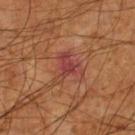notes: total-body-photography surveillance lesion; no biopsy
subject: male, aged 58 to 62
image: ~15 mm crop, total-body skin-cancer survey
site: the left lower leg